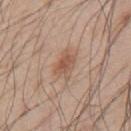Findings:
• biopsy status · total-body-photography surveillance lesion; no biopsy
• anatomic site · the upper back
• image source · total-body-photography crop, ~15 mm field of view
• lighting · white-light illumination
• patient · male, approximately 55 years of age
• lesion diameter · about 3.5 mm
• image-analysis metrics · a border-irregularity rating of about 3.5/10, a color-variation rating of about 2.5/10, and peripheral color asymmetry of about 1; an automated nevus-likeness rating near 75 out of 100 and lesion-presence confidence of about 100/100